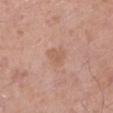  biopsy_status: not biopsied; imaged during a skin examination
  lighting: white-light
  patient:
    sex: male
    age_approx: 80
  image:
    source: total-body photography crop
    field_of_view_mm: 15
  lesion_size:
    long_diameter_mm_approx: 2.5
  site: leg